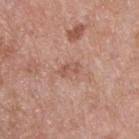* workup: total-body-photography surveillance lesion; no biopsy
* size: about 2.5 mm
* image source: ~15 mm tile from a whole-body skin photo
* patient: male, in their 50s
* location: the front of the torso